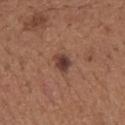The lesion was photographed on a routine skin check and not biopsied; there is no pathology result. This is a white-light tile. The lesion-visualizer software estimated a lesion area of about 4.5 mm², a shape eccentricity near 0.55, and two-axis asymmetry of about 0.3. The software also gave a lesion-to-skin contrast of about 9.5 (normalized; higher = more distinct). It also reported a border-irregularity rating of about 2.5/10 and internal color variation of about 3.5 on a 0–10 scale. A close-up tile cropped from a whole-body skin photograph, about 15 mm across. Measured at roughly 2.5 mm in maximum diameter. The lesion is located on the mid back. A male patient about 65 years old.